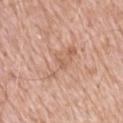This is a white-light tile. Automated image analysis of the tile measured an area of roughly 11 mm², a shape eccentricity near 0.8, and a symmetry-axis asymmetry near 0.35. The software also gave border irregularity of about 6 on a 0–10 scale and a color-variation rating of about 3/10. A male patient in their 50s. A 15 mm close-up extracted from a 3D total-body photography capture. The lesion is on the mid back.